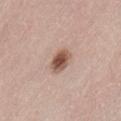follow-up=no biopsy performed (imaged during a skin exam)
image source=total-body-photography crop, ~15 mm field of view
subject=female, aged around 65
anatomic site=the lower back
diameter=~3 mm (longest diameter)